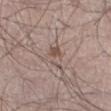notes — catalogued during a skin exam; not biopsied
lighting — white-light
subject — male, aged around 55
automated metrics — an area of roughly 6.5 mm², an outline eccentricity of about 0.9 (0 = round, 1 = elongated), and two-axis asymmetry of about 0.4; a nevus-likeness score of about 0/100 and lesion-presence confidence of about 95/100
acquisition — total-body-photography crop, ~15 mm field of view
body site — the right thigh